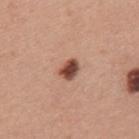{"biopsy_status": "not biopsied; imaged during a skin examination", "lesion_size": {"long_diameter_mm_approx": 2.5}, "patient": {"sex": "male", "age_approx": 40}, "site": "upper back", "image": {"source": "total-body photography crop", "field_of_view_mm": 15}}